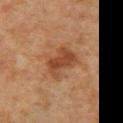Imaged during a routine full-body skin examination; the lesion was not biopsied and no histopathology is available.